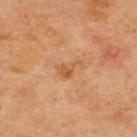- biopsy status · imaged on a skin check; not biopsied
- site · the upper back
- illumination · cross-polarized
- patient · male, aged 68 to 72
- image-analysis metrics · an area of roughly 4 mm² and an outline eccentricity of about 0.85 (0 = round, 1 = elongated); an average lesion color of about L≈55 a*≈23 b*≈40 (CIELAB) and a lesion–skin lightness drop of about 7; border irregularity of about 6 on a 0–10 scale, internal color variation of about 2 on a 0–10 scale, and a peripheral color-asymmetry measure near 1
- lesion size · ≈3 mm
- image · 15 mm crop, total-body photography